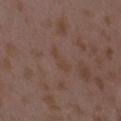<record>
  <biopsy_status>not biopsied; imaged during a skin examination</biopsy_status>
  <lesion_size>
    <long_diameter_mm_approx>3.5</long_diameter_mm_approx>
  </lesion_size>
  <automated_metrics>
    <cielab_L>42</cielab_L>
    <cielab_a>16</cielab_a>
    <cielab_b>24</cielab_b>
    <vs_skin_darker_L>4.0</vs_skin_darker_L>
    <vs_skin_contrast_norm>4.5</vs_skin_contrast_norm>
    <nevus_likeness_0_100>0</nevus_likeness_0_100>
    <lesion_detection_confidence_0_100>100</lesion_detection_confidence_0_100>
  </automated_metrics>
  <patient>
    <sex>female</sex>
    <age_approx>35</age_approx>
  </patient>
  <image>
    <source>total-body photography crop</source>
    <field_of_view_mm>15</field_of_view_mm>
  </image>
  <site>arm</site>
  <lighting>white-light</lighting>
</record>